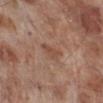Background:
An algorithmic analysis of the crop reported a lesion–skin lightness drop of about 7 and a normalized lesion–skin contrast near 6. The analysis additionally found border irregularity of about 4 on a 0–10 scale and internal color variation of about 1.5 on a 0–10 scale. And it measured a nevus-likeness score of about 0/100 and a lesion-detection confidence of about 100/100. A male subject aged 68 to 72. The lesion is located on the right lower leg. Cropped from a total-body skin-imaging series; the visible field is about 15 mm. The recorded lesion diameter is about 3.5 mm. Imaged with white-light lighting.